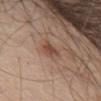Part of a total-body skin-imaging series; this lesion was reviewed on a skin check and was not flagged for biopsy. A male subject aged 63–67. From the right upper arm. Approximately 2.5 mm at its widest. The tile uses white-light illumination. An algorithmic analysis of the crop reported a lesion area of about 3.5 mm² and a shape-asymmetry score of about 0.3 (0 = symmetric). And it measured about 9 CIELAB-L* units darker than the surrounding skin and a lesion-to-skin contrast of about 7.5 (normalized; higher = more distinct). The analysis additionally found a nevus-likeness score of about 40/100. A 15 mm close-up tile from a total-body photography series done for melanoma screening.